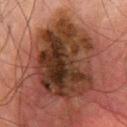| field | value |
|---|---|
| notes | imaged on a skin check; not biopsied |
| imaging modality | total-body-photography crop, ~15 mm field of view |
| subject | male, aged around 65 |
| lesion diameter | ~9.5 mm (longest diameter) |
| body site | the upper back |
| tile lighting | cross-polarized |